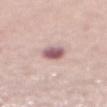This lesion was catalogued during total-body skin photography and was not selected for biopsy. A 15 mm crop from a total-body photograph taken for skin-cancer surveillance. On the front of the torso. A female patient, in their 70s.